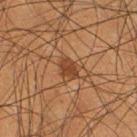notes = no biopsy performed (imaged during a skin exam); anatomic site = the right thigh; image source = 15 mm crop, total-body photography; subject = male, aged approximately 50.